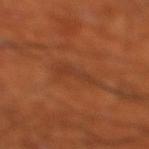This lesion was catalogued during total-body skin photography and was not selected for biopsy. The lesion is located on the right thigh. The tile uses cross-polarized illumination. The patient is a male aged around 70. A close-up tile cropped from a whole-body skin photograph, about 15 mm across. The lesion-visualizer software estimated an automated nevus-likeness rating near 0 out of 100 and a lesion-detection confidence of about 55/100.